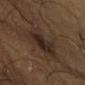No biopsy was performed on this lesion — it was imaged during a full skin examination and was not determined to be concerning.